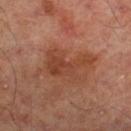Part of a total-body skin-imaging series; this lesion was reviewed on a skin check and was not flagged for biopsy. A male subject, roughly 50 years of age. A 15 mm close-up extracted from a 3D total-body photography capture. Automated tile analysis of the lesion measured a mean CIELAB color near L≈42 a*≈25 b*≈32, about 8 CIELAB-L* units darker than the surrounding skin, and a lesion-to-skin contrast of about 7 (normalized; higher = more distinct). It also reported a detector confidence of about 100 out of 100 that the crop contains a lesion. The lesion is on the left leg. This is a cross-polarized tile. Approximately 5.5 mm at its widest.